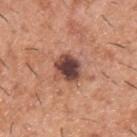This lesion was catalogued during total-body skin photography and was not selected for biopsy. The recorded lesion diameter is about 3 mm. The total-body-photography lesion software estimated a classifier nevus-likeness of about 15/100 and a lesion-detection confidence of about 100/100. From the upper back. The subject is a male about 40 years old. Cropped from a total-body skin-imaging series; the visible field is about 15 mm. Imaged with white-light lighting.